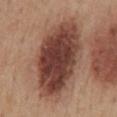Part of a total-body skin-imaging series; this lesion was reviewed on a skin check and was not flagged for biopsy.
A 15 mm close-up tile from a total-body photography series done for melanoma screening.
From the mid back.
Approximately 10.5 mm at its widest.
A male patient, approximately 55 years of age.